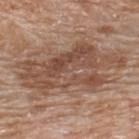Image and clinical context: The subject is a male aged 78 to 82. A roughly 15 mm field-of-view crop from a total-body skin photograph. The lesion is located on the upper back. The total-body-photography lesion software estimated a footprint of about 43 mm² and a shape-asymmetry score of about 0.35 (0 = symmetric).